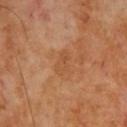Imaged during a routine full-body skin examination; the lesion was not biopsied and no histopathology is available. Captured under cross-polarized illumination. A male subject, aged approximately 65. Approximately 3 mm at its widest. Cropped from a whole-body photographic skin survey; the tile spans about 15 mm.